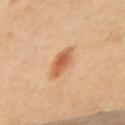Clinical impression: Captured during whole-body skin photography for melanoma surveillance; the lesion was not biopsied. Clinical summary: A lesion tile, about 15 mm wide, cut from a 3D total-body photograph. This is a cross-polarized tile. The recorded lesion diameter is about 4 mm. The lesion is located on the upper back.